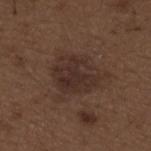Q: What kind of image is this?
A: total-body-photography crop, ~15 mm field of view
Q: Lesion location?
A: the back
Q: Illumination type?
A: white-light illumination
Q: Who is the patient?
A: male, about 50 years old
Q: Lesion size?
A: about 5.5 mm